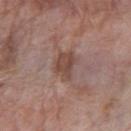Clinical impression:
Captured during whole-body skin photography for melanoma surveillance; the lesion was not biopsied.
Clinical summary:
A female patient aged 68 to 72. Located on the right forearm. The tile uses white-light illumination. Measured at roughly 4 mm in maximum diameter. Automated image analysis of the tile measured a color-variation rating of about 2.5/10 and a peripheral color-asymmetry measure near 1. A 15 mm crop from a total-body photograph taken for skin-cancer surveillance.